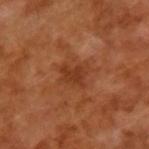Clinical impression: No biopsy was performed on this lesion — it was imaged during a full skin examination and was not determined to be concerning. Image and clinical context: A male subject aged around 65. The recorded lesion diameter is about 3 mm. An algorithmic analysis of the crop reported an average lesion color of about L≈37 a*≈26 b*≈34 (CIELAB), about 8 CIELAB-L* units darker than the surrounding skin, and a normalized border contrast of about 6.5. The software also gave a within-lesion color-variation index near 2.5/10 and radial color variation of about 1. The software also gave a detector confidence of about 100 out of 100 that the crop contains a lesion. A close-up tile cropped from a whole-body skin photograph, about 15 mm across.